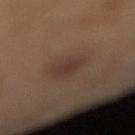Clinical impression: This lesion was catalogued during total-body skin photography and was not selected for biopsy. Clinical summary: Automated tile analysis of the lesion measured a footprint of about 7.5 mm², an outline eccentricity of about 0.5 (0 = round, 1 = elongated), and two-axis asymmetry of about 0.2. And it measured a lesion color around L≈36 a*≈15 b*≈24 in CIELAB, roughly 7 lightness units darker than nearby skin, and a normalized lesion–skin contrast near 6.5. The software also gave border irregularity of about 2 on a 0–10 scale and internal color variation of about 2 on a 0–10 scale. The subject is a female in their mid- to late 50s. This is a cross-polarized tile. Cropped from a total-body skin-imaging series; the visible field is about 15 mm. From the right lower leg.